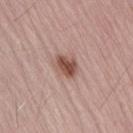Recorded during total-body skin imaging; not selected for excision or biopsy. A roughly 15 mm field-of-view crop from a total-body skin photograph. On the lower back. A male patient aged approximately 70.